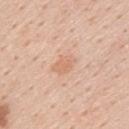Notes:
• notes: total-body-photography surveillance lesion; no biopsy
• site: the upper back
• TBP lesion metrics: an area of roughly 4.5 mm², an eccentricity of roughly 0.75, and a shape-asymmetry score of about 0.25 (0 = symmetric); an average lesion color of about L≈68 a*≈21 b*≈33 (CIELAB) and about 7 CIELAB-L* units darker than the surrounding skin; a nevus-likeness score of about 0/100 and lesion-presence confidence of about 100/100
• size: ≈3 mm
• patient: male, approximately 60 years of age
• image source: ~15 mm tile from a whole-body skin photo